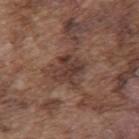The lesion was tiled from a total-body skin photograph and was not biopsied. About 3.5 mm across. The lesion is located on the mid back. This is a white-light tile. A roughly 15 mm field-of-view crop from a total-body skin photograph. The subject is a male in their mid-70s.